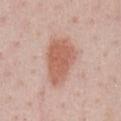Q: Is there a histopathology result?
A: catalogued during a skin exam; not biopsied
Q: Patient demographics?
A: male, aged around 60
Q: Lesion location?
A: the chest
Q: What is the imaging modality?
A: ~15 mm tile from a whole-body skin photo